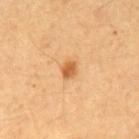Q: Lesion location?
A: the mid back
Q: What is the imaging modality?
A: 15 mm crop, total-body photography
Q: What are the patient's age and sex?
A: male, approximately 60 years of age